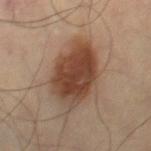biopsy_status: not biopsied; imaged during a skin examination
site: right thigh
lighting: cross-polarized
image:
  source: total-body photography crop
  field_of_view_mm: 15